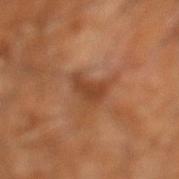Q: Who is the patient?
A: male, aged around 60
Q: What lighting was used for the tile?
A: cross-polarized
Q: What is the imaging modality?
A: ~15 mm tile from a whole-body skin photo
Q: How large is the lesion?
A: ~4 mm (longest diameter)
Q: What is the anatomic site?
A: the right leg